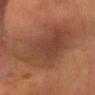Part of a total-body skin-imaging series; this lesion was reviewed on a skin check and was not flagged for biopsy. Captured under cross-polarized illumination. The patient is a male roughly 65 years of age. Longest diameter approximately 9.5 mm. A roughly 15 mm field-of-view crop from a total-body skin photograph. On the head or neck.